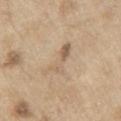Impression: No biopsy was performed on this lesion — it was imaged during a full skin examination and was not determined to be concerning. Acquisition and patient details: The total-body-photography lesion software estimated a shape-asymmetry score of about 0.45 (0 = symmetric). It also reported an average lesion color of about L≈61 a*≈14 b*≈31 (CIELAB), a lesion–skin lightness drop of about 8, and a normalized border contrast of about 5. And it measured internal color variation of about 4 on a 0–10 scale and a peripheral color-asymmetry measure near 1. A male subject aged approximately 70. Cropped from a whole-body photographic skin survey; the tile spans about 15 mm. The lesion is on the left upper arm.